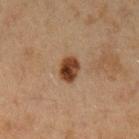notes: total-body-photography surveillance lesion; no biopsy
location: the left upper arm
automated metrics: a mean CIELAB color near L≈35 a*≈19 b*≈29, roughly 15 lightness units darker than nearby skin, and a lesion-to-skin contrast of about 12.5 (normalized; higher = more distinct); a nevus-likeness score of about 100/100 and a lesion-detection confidence of about 100/100
illumination: cross-polarized
patient: female, aged around 20
image source: ~15 mm tile from a whole-body skin photo
size: ~3 mm (longest diameter)